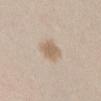A female subject, aged approximately 25. The lesion is located on the front of the torso. A lesion tile, about 15 mm wide, cut from a 3D total-body photograph.